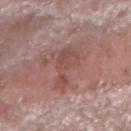Q: Was a biopsy performed?
A: total-body-photography surveillance lesion; no biopsy
Q: What is the imaging modality?
A: ~15 mm crop, total-body skin-cancer survey
Q: Who is the patient?
A: female, about 65 years old
Q: Automated lesion metrics?
A: a lesion area of about 8.5 mm², a shape eccentricity near 0.85, and two-axis asymmetry of about 0.55; a nevus-likeness score of about 0/100 and lesion-presence confidence of about 100/100
Q: What is the lesion's diameter?
A: ≈5 mm
Q: What lighting was used for the tile?
A: white-light illumination
Q: Lesion location?
A: the arm